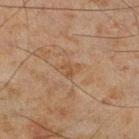Case summary:
– workup — total-body-photography surveillance lesion; no biopsy
– image source — ~15 mm tile from a whole-body skin photo
– lesion size — ≈2.5 mm
– subject — male, in their mid- to late 40s
– location — the right lower leg
– lighting — cross-polarized